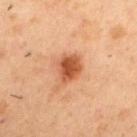  biopsy_status: not biopsied; imaged during a skin examination
  lesion_size:
    long_diameter_mm_approx: 3.5
  patient:
    sex: male
    age_approx: 55
  site: upper back
  image:
    source: total-body photography crop
    field_of_view_mm: 15
  lighting: cross-polarized